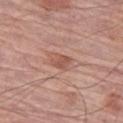The lesion was tiled from a total-body skin photograph and was not biopsied.
This image is a 15 mm lesion crop taken from a total-body photograph.
From the left thigh.
A male subject aged approximately 75.
The lesion's longest dimension is about 2.5 mm.
Imaged with white-light lighting.